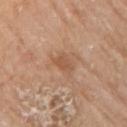<record>
  <biopsy_status>not biopsied; imaged during a skin examination</biopsy_status>
  <lighting>white-light</lighting>
  <site>right upper arm</site>
  <lesion_size>
    <long_diameter_mm_approx>3.0</long_diameter_mm_approx>
  </lesion_size>
  <image>
    <source>total-body photography crop</source>
    <field_of_view_mm>15</field_of_view_mm>
  </image>
  <patient>
    <sex>male</sex>
    <age_approx>80</age_approx>
  </patient>
</record>